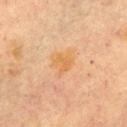Case summary:
• follow-up · total-body-photography surveillance lesion; no biopsy
• anatomic site · the chest
• lesion diameter · ~2.5 mm (longest diameter)
• image source · ~15 mm crop, total-body skin-cancer survey
• lighting · cross-polarized
• TBP lesion metrics · an average lesion color of about L≈58 a*≈21 b*≈40 (CIELAB), roughly 5 lightness units darker than nearby skin, and a normalized border contrast of about 6.5; a color-variation rating of about 1.5/10 and radial color variation of about 0.5; a classifier nevus-likeness of about 20/100 and a lesion-detection confidence of about 100/100
• patient · female, roughly 60 years of age